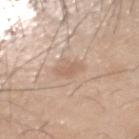{
  "automated_metrics": {
    "cielab_L": 62,
    "cielab_a": 17,
    "cielab_b": 29,
    "vs_skin_darker_L": 7.0,
    "vs_skin_contrast_norm": 5.0,
    "border_irregularity_0_10": 3.0,
    "color_variation_0_10": 1.0,
    "peripheral_color_asymmetry": 0.5
  },
  "lighting": "white-light",
  "site": "head or neck",
  "image": {
    "source": "total-body photography crop",
    "field_of_view_mm": 15
  },
  "patient": {
    "sex": "male",
    "age_approx": 45
  }
}